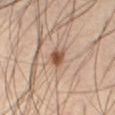The lesion was tiled from a total-body skin photograph and was not biopsied. From the front of the torso. A 15 mm crop from a total-body photograph taken for skin-cancer surveillance. The subject is a male in their 60s. The recorded lesion diameter is about 2 mm.